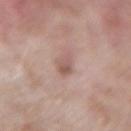Captured during whole-body skin photography for melanoma surveillance; the lesion was not biopsied.
A male subject aged 58 to 62.
On the left forearm.
A 15 mm close-up extracted from a 3D total-body photography capture.
The tile uses white-light illumination.
Longest diameter approximately 3 mm.
An algorithmic analysis of the crop reported a footprint of about 4 mm², an eccentricity of roughly 0.85, and a shape-asymmetry score of about 0.25 (0 = symmetric). And it measured a lesion color around L≈56 a*≈19 b*≈23 in CIELAB and a lesion–skin lightness drop of about 9. And it measured a border-irregularity rating of about 2.5/10 and a peripheral color-asymmetry measure near 1.